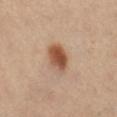Located on the right leg.
A 15 mm close-up tile from a total-body photography series done for melanoma screening.
This is a cross-polarized tile.
A female patient, aged approximately 40.
The lesion's longest dimension is about 3.5 mm.